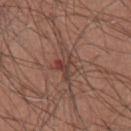Assessment:
No biopsy was performed on this lesion — it was imaged during a full skin examination and was not determined to be concerning.
Context:
Imaged with white-light lighting. Automated tile analysis of the lesion measured an area of roughly 7 mm² and a shape eccentricity near 0.85. It also reported an average lesion color of about L≈42 a*≈20 b*≈24 (CIELAB), a lesion–skin lightness drop of about 8, and a normalized border contrast of about 6.5. And it measured border irregularity of about 5.5 on a 0–10 scale, a within-lesion color-variation index near 8.5/10, and peripheral color asymmetry of about 3.5. It also reported a nevus-likeness score of about 0/100. The lesion is located on the front of the torso. A roughly 15 mm field-of-view crop from a total-body skin photograph. A male subject roughly 60 years of age. The recorded lesion diameter is about 4.5 mm.